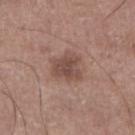This lesion was catalogued during total-body skin photography and was not selected for biopsy. Captured under white-light illumination. A lesion tile, about 15 mm wide, cut from a 3D total-body photograph. Automated image analysis of the tile measured a footprint of about 8.5 mm² and a shape eccentricity near 0.65. The subject is a male in their mid-60s. The lesion is located on the leg.